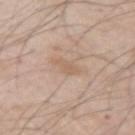Assessment:
The lesion was photographed on a routine skin check and not biopsied; there is no pathology result.
Context:
A male patient, aged around 45. A roughly 15 mm field-of-view crop from a total-body skin photograph. On the arm.